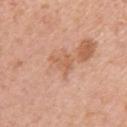Imaged during a routine full-body skin examination; the lesion was not biopsied and no histopathology is available. Located on the right upper arm. A female subject, aged 38–42. A close-up tile cropped from a whole-body skin photograph, about 15 mm across. Captured under white-light illumination. The recorded lesion diameter is about 3 mm.